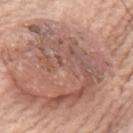biopsy status=imaged on a skin check; not biopsied | site=the chest | patient=male, aged 78–82 | acquisition=15 mm crop, total-body photography | illumination=white-light.